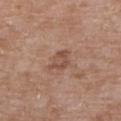No biopsy was performed on this lesion — it was imaged during a full skin examination and was not determined to be concerning. Automated image analysis of the tile measured an average lesion color of about L≈50 a*≈20 b*≈28 (CIELAB), a lesion–skin lightness drop of about 8, and a lesion-to-skin contrast of about 6 (normalized; higher = more distinct). The software also gave an automated nevus-likeness rating near 0 out of 100 and a lesion-detection confidence of about 100/100. A 15 mm close-up tile from a total-body photography series done for melanoma screening. Captured under white-light illumination. The recorded lesion diameter is about 3 mm. On the upper back. The patient is a female roughly 65 years of age.